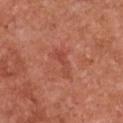workup: total-body-photography surveillance lesion; no biopsy | anatomic site: the chest | tile lighting: white-light illumination | patient: female, aged around 50 | lesion diameter: ~3.5 mm (longest diameter) | image: 15 mm crop, total-body photography | automated lesion analysis: lesion-presence confidence of about 100/100.